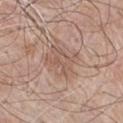  biopsy_status: not biopsied; imaged during a skin examination
  patient:
    sex: male
    age_approx: 65
  lesion_size:
    long_diameter_mm_approx: 4.0
  lighting: white-light
  automated_metrics:
    eccentricity: 0.4
    border_irregularity_0_10: 3.0
    color_variation_0_10: 3.5
    peripheral_color_asymmetry: 1.0
    nevus_likeness_0_100: 0
    lesion_detection_confidence_0_100: 100
  site: chest
  image:
    source: total-body photography crop
    field_of_view_mm: 15